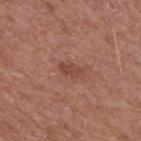Imaged during a routine full-body skin examination; the lesion was not biopsied and no histopathology is available.
Longest diameter approximately 2.5 mm.
The lesion is on the back.
The subject is a male approximately 65 years of age.
A lesion tile, about 15 mm wide, cut from a 3D total-body photograph.
The tile uses white-light illumination.
The total-body-photography lesion software estimated an average lesion color of about L≈45 a*≈24 b*≈27 (CIELAB).